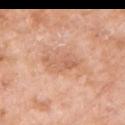Findings:
* follow-up · imaged on a skin check; not biopsied
* image source · ~15 mm tile from a whole-body skin photo
* patient · female, aged 73–77
* location · the left upper arm
* size · about 4.5 mm
* automated lesion analysis · a lesion area of about 6.5 mm², a shape eccentricity near 0.9, and two-axis asymmetry of about 0.45; a lesion color around L≈62 a*≈23 b*≈33 in CIELAB and a lesion–skin lightness drop of about 9